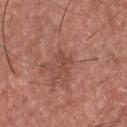workup: no biopsy performed (imaged during a skin exam); acquisition: 15 mm crop, total-body photography; anatomic site: the upper back; subject: male, about 45 years old.